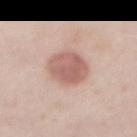Recorded during total-body skin imaging; not selected for excision or biopsy. A male subject, roughly 40 years of age. From the front of the torso. This image is a 15 mm lesion crop taken from a total-body photograph.